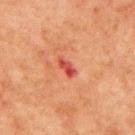Q: What is the lesion's diameter?
A: ≈3 mm
Q: Patient demographics?
A: male, about 80 years old
Q: Automated lesion metrics?
A: a mean CIELAB color near L≈41 a*≈33 b*≈29 and a lesion-to-skin contrast of about 8 (normalized; higher = more distinct); a classifier nevus-likeness of about 0/100 and a lesion-detection confidence of about 100/100
Q: What is the anatomic site?
A: the mid back
Q: What is the imaging modality?
A: ~15 mm tile from a whole-body skin photo